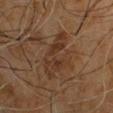Recorded during total-body skin imaging; not selected for excision or biopsy. The recorded lesion diameter is about 6.5 mm. A male subject, aged 58 to 62. A 15 mm close-up tile from a total-body photography series done for melanoma screening. Captured under cross-polarized illumination. An algorithmic analysis of the crop reported a border-irregularity index near 4.5/10, a color-variation rating of about 4/10, and a peripheral color-asymmetry measure near 1.5. It also reported a nevus-likeness score of about 0/100 and lesion-presence confidence of about 95/100. Located on the front of the torso.